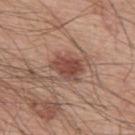Recorded during total-body skin imaging; not selected for excision or biopsy. A male subject roughly 55 years of age. A 15 mm crop from a total-body photograph taken for skin-cancer surveillance. From the upper back.